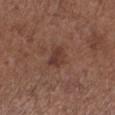The lesion was photographed on a routine skin check and not biopsied; there is no pathology result. The lesion is on the left lower leg. A female patient, roughly 55 years of age. The lesion-visualizer software estimated an area of roughly 4.5 mm², a shape eccentricity near 0.6, and a shape-asymmetry score of about 0.25 (0 = symmetric). It also reported a mean CIELAB color near L≈37 a*≈20 b*≈24, about 8 CIELAB-L* units darker than the surrounding skin, and a normalized border contrast of about 7. And it measured a border-irregularity rating of about 2.5/10 and a peripheral color-asymmetry measure near 1. It also reported lesion-presence confidence of about 100/100. Longest diameter approximately 2.5 mm. Captured under white-light illumination. Cropped from a total-body skin-imaging series; the visible field is about 15 mm.